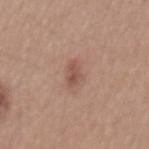Impression:
The lesion was tiled from a total-body skin photograph and was not biopsied.
Background:
Captured under white-light illumination. Longest diameter approximately 3 mm. From the mid back. Automated tile analysis of the lesion measured a lesion–skin lightness drop of about 9 and a normalized lesion–skin contrast near 6.5. The software also gave a color-variation rating of about 2.5/10 and peripheral color asymmetry of about 1. Cropped from a whole-body photographic skin survey; the tile spans about 15 mm. The subject is a male in their mid- to late 50s.